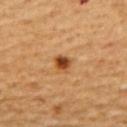Clinical impression:
The lesion was photographed on a routine skin check and not biopsied; there is no pathology result.
Context:
Cropped from a whole-body photographic skin survey; the tile spans about 15 mm. The lesion is located on the upper back. The lesion's longest dimension is about 2 mm. Captured under cross-polarized illumination. A male subject, aged around 60.